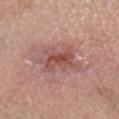Background: A male subject, in their 70s. About 5.5 mm across. Cropped from a whole-body photographic skin survey; the tile spans about 15 mm. The lesion is located on the head or neck. Pathology: The biopsy diagnosis was a malignant lesion: invasive squamous cell carcinoma.